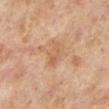workup: no biopsy performed (imaged during a skin exam) | patient: female, roughly 40 years of age | anatomic site: the left lower leg | image source: ~15 mm crop, total-body skin-cancer survey | tile lighting: cross-polarized | lesion size: about 3.5 mm.